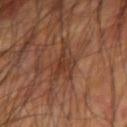Captured during whole-body skin photography for melanoma surveillance; the lesion was not biopsied. A male subject aged 58 to 62. This is a cross-polarized tile. Measured at roughly 5 mm in maximum diameter. The lesion-visualizer software estimated about 7 CIELAB-L* units darker than the surrounding skin and a normalized lesion–skin contrast near 6. The lesion is located on the left upper arm. A roughly 15 mm field-of-view crop from a total-body skin photograph.